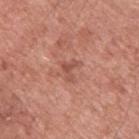biopsy_status: not biopsied; imaged during a skin examination
lighting: white-light
patient:
  sex: male
  age_approx: 55
automated_metrics:
  area_mm2_approx: 3.0
  shape_asymmetry: 0.65
  nevus_likeness_0_100: 0
  lesion_detection_confidence_0_100: 100
site: upper back
image:
  source: total-body photography crop
  field_of_view_mm: 15